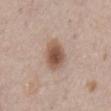Notes:
• subject · male, aged 63–67
• diameter · ~4 mm (longest diameter)
• automated lesion analysis · about 14 CIELAB-L* units darker than the surrounding skin; a border-irregularity rating of about 1.5/10; a nevus-likeness score of about 100/100
• image · ~15 mm tile from a whole-body skin photo
• anatomic site · the chest
• lighting · white-light